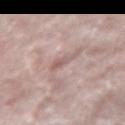Located on the right forearm. Captured under white-light illumination. A female subject approximately 50 years of age. Measured at roughly 3 mm in maximum diameter. Cropped from a whole-body photographic skin survey; the tile spans about 15 mm.